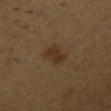Part of a total-body skin-imaging series; this lesion was reviewed on a skin check and was not flagged for biopsy.
Approximately 3.5 mm at its widest.
A roughly 15 mm field-of-view crop from a total-body skin photograph.
The subject is a female in their 40s.
The tile uses cross-polarized illumination.
Automated image analysis of the tile measured a peripheral color-asymmetry measure near 0.5. The analysis additionally found a nevus-likeness score of about 80/100 and lesion-presence confidence of about 100/100.
From the chest.